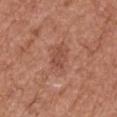Assessment:
Part of a total-body skin-imaging series; this lesion was reviewed on a skin check and was not flagged for biopsy.
Image and clinical context:
A male patient, aged approximately 75. A roughly 15 mm field-of-view crop from a total-body skin photograph. Longest diameter approximately 3 mm. The tile uses white-light illumination. The lesion is on the arm. Automated tile analysis of the lesion measured a footprint of about 4 mm², an outline eccentricity of about 0.8 (0 = round, 1 = elongated), and a shape-asymmetry score of about 0.25 (0 = symmetric). The software also gave an average lesion color of about L≈49 a*≈25 b*≈29 (CIELAB) and a normalized border contrast of about 5.5. The analysis additionally found a classifier nevus-likeness of about 0/100 and a lesion-detection confidence of about 100/100.